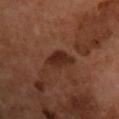Impression:
Recorded during total-body skin imaging; not selected for excision or biopsy.
Image and clinical context:
Cropped from a total-body skin-imaging series; the visible field is about 15 mm. On the chest. A male patient in their mid-60s. Automated tile analysis of the lesion measured an area of roughly 5.5 mm², an outline eccentricity of about 0.85 (0 = round, 1 = elongated), and a symmetry-axis asymmetry near 0.3. And it measured a classifier nevus-likeness of about 55/100 and a lesion-detection confidence of about 100/100.